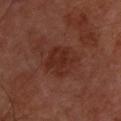{
  "patient": {
    "sex": "male",
    "age_approx": 50
  },
  "image": {
    "source": "total-body photography crop",
    "field_of_view_mm": 15
  },
  "site": "upper back",
  "lighting": "cross-polarized"
}